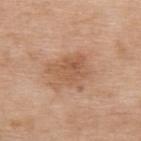Captured during whole-body skin photography for melanoma surveillance; the lesion was not biopsied. A female patient, roughly 75 years of age. Captured under white-light illumination. Cropped from a whole-body photographic skin survey; the tile spans about 15 mm. Approximately 4.5 mm at its widest. The lesion is on the upper back.